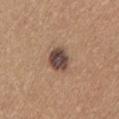No biopsy was performed on this lesion — it was imaged during a full skin examination and was not determined to be concerning.
Captured under white-light illumination.
The lesion is on the lower back.
Cropped from a total-body skin-imaging series; the visible field is about 15 mm.
A male subject, in their mid- to late 60s.
Longest diameter approximately 3.5 mm.